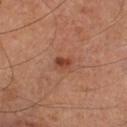Impression:
Captured during whole-body skin photography for melanoma surveillance; the lesion was not biopsied.
Background:
The lesion-visualizer software estimated a lesion color around L≈41 a*≈25 b*≈30 in CIELAB, roughly 10 lightness units darker than nearby skin, and a normalized lesion–skin contrast near 8. The software also gave a border-irregularity index near 1.5/10 and a color-variation rating of about 2.5/10. A male patient approximately 65 years of age. About 2 mm across. The lesion is located on the left lower leg. Captured under cross-polarized illumination. This image is a 15 mm lesion crop taken from a total-body photograph.